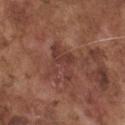Q: Was a biopsy performed?
A: total-body-photography surveillance lesion; no biopsy
Q: How was this image acquired?
A: 15 mm crop, total-body photography
Q: Lesion location?
A: the front of the torso
Q: What are the patient's age and sex?
A: male, approximately 75 years of age